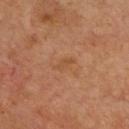workup — no biopsy performed (imaged during a skin exam) | TBP lesion metrics — a footprint of about 3 mm², an outline eccentricity of about 0.9 (0 = round, 1 = elongated), and a shape-asymmetry score of about 0.35 (0 = symmetric); a lesion color around L≈50 a*≈22 b*≈36 in CIELAB, a lesion–skin lightness drop of about 5, and a lesion-to-skin contrast of about 5 (normalized; higher = more distinct) | patient — male, about 50 years old | diameter — ≈3 mm | image — 15 mm crop, total-body photography | anatomic site — the chest | lighting — cross-polarized illumination.